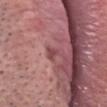notes: no biopsy performed (imaged during a skin exam); lighting: white-light illumination; acquisition: 15 mm crop, total-body photography; anatomic site: the head or neck; patient: male, aged around 60.